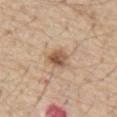The lesion was photographed on a routine skin check and not biopsied; there is no pathology result. The tile uses white-light illumination. A male patient, aged approximately 70. On the abdomen. A 15 mm close-up tile from a total-body photography series done for melanoma screening. The lesion's longest dimension is about 2.5 mm.